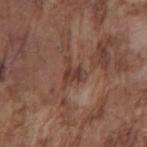Captured during whole-body skin photography for melanoma surveillance; the lesion was not biopsied.
The subject is a male in their mid-70s.
The lesion is on the mid back.
This is a white-light tile.
Automated image analysis of the tile measured a lesion area of about 5 mm², an eccentricity of roughly 0.5, and two-axis asymmetry of about 0.6.
Cropped from a total-body skin-imaging series; the visible field is about 15 mm.